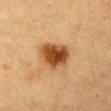The tile uses cross-polarized illumination.
Cropped from a total-body skin-imaging series; the visible field is about 15 mm.
A female subject in their mid-50s.
The lesion is located on the chest.
The lesion's longest dimension is about 4.5 mm.